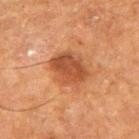workup: imaged on a skin check; not biopsied | site: the right upper arm | lesion diameter: about 5 mm | TBP lesion metrics: a mean CIELAB color near L≈41 a*≈23 b*≈32 and a normalized lesion–skin contrast near 8 | image: ~15 mm crop, total-body skin-cancer survey | patient: male, aged 73 to 77.